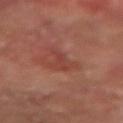workup: no biopsy performed (imaged during a skin exam); illumination: cross-polarized; body site: the arm; image: 15 mm crop, total-body photography; lesion size: ~3 mm (longest diameter); subject: male, approximately 65 years of age.